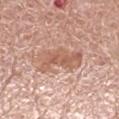Impression: This lesion was catalogued during total-body skin photography and was not selected for biopsy. Clinical summary: The tile uses white-light illumination. The lesion is located on the right lower leg. The patient is a female aged around 50. A close-up tile cropped from a whole-body skin photograph, about 15 mm across.